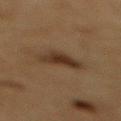| field | value |
|---|---|
| biopsy status | total-body-photography surveillance lesion; no biopsy |
| image-analysis metrics | a nevus-likeness score of about 90/100 and a detector confidence of about 100 out of 100 that the crop contains a lesion |
| patient | male, in their mid- to late 80s or older |
| body site | the back |
| diameter | about 4 mm |
| acquisition | total-body-photography crop, ~15 mm field of view |